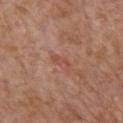Clinical impression:
The lesion was tiled from a total-body skin photograph and was not biopsied.
Context:
A male patient, in their mid-60s. The tile uses white-light illumination. The lesion's longest dimension is about 2.5 mm. Located on the chest. Cropped from a total-body skin-imaging series; the visible field is about 15 mm.